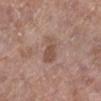biopsy status: catalogued during a skin exam; not biopsied
subject: female, aged 73–77
illumination: white-light
site: the left lower leg
imaging modality: 15 mm crop, total-body photography
TBP lesion metrics: a footprint of about 7.5 mm² and an outline eccentricity of about 0.8 (0 = round, 1 = elongated); a classifier nevus-likeness of about 0/100